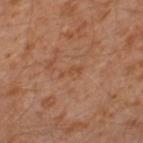biopsy_status: not biopsied; imaged during a skin examination
patient:
  sex: male
  age_approx: 30
lighting: cross-polarized
site: left thigh
image:
  source: total-body photography crop
  field_of_view_mm: 15